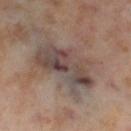Notes:
– biopsy status · imaged on a skin check; not biopsied
– acquisition · total-body-photography crop, ~15 mm field of view
– image-analysis metrics · a lesion area of about 28 mm² and an eccentricity of roughly 0.75; an average lesion color of about L≈46 a*≈13 b*≈20 (CIELAB) and a normalized lesion–skin contrast near 8.5; a border-irregularity rating of about 4.5/10, internal color variation of about 9.5 on a 0–10 scale, and peripheral color asymmetry of about 3.5; a nevus-likeness score of about 0/100 and a lesion-detection confidence of about 70/100
– subject · female, aged around 55
– lesion size · about 7.5 mm
– tile lighting · cross-polarized
– anatomic site · the right thigh